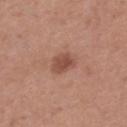Recorded during total-body skin imaging; not selected for excision or biopsy.